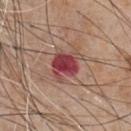follow-up=total-body-photography surveillance lesion; no biopsy
site=the chest
image=total-body-photography crop, ~15 mm field of view
diameter=about 4 mm
subject=male, approximately 65 years of age
tile lighting=white-light illumination